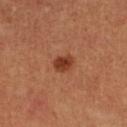biopsy status=imaged on a skin check; not biopsied
location=the right lower leg
patient=female
automated metrics=a lesion area of about 4.5 mm² and two-axis asymmetry of about 0.2; a lesion–skin lightness drop of about 12 and a normalized lesion–skin contrast near 9; border irregularity of about 1.5 on a 0–10 scale, internal color variation of about 2.5 on a 0–10 scale, and radial color variation of about 1
diameter=≈2.5 mm
lighting=cross-polarized illumination
image=~15 mm tile from a whole-body skin photo